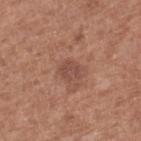Imaged with white-light lighting. From the right lower leg. An algorithmic analysis of the crop reported a footprint of about 5.5 mm² and a shape-asymmetry score of about 0.2 (0 = symmetric). And it measured a lesion color around L≈48 a*≈22 b*≈26 in CIELAB and a normalized border contrast of about 6. It also reported a detector confidence of about 100 out of 100 that the crop contains a lesion. The subject is a male in their mid-70s. Cropped from a total-body skin-imaging series; the visible field is about 15 mm.